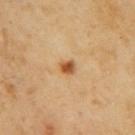Assessment: Imaged during a routine full-body skin examination; the lesion was not biopsied and no histopathology is available. Image and clinical context: A lesion tile, about 15 mm wide, cut from a 3D total-body photograph. The lesion's longest dimension is about 2 mm. Automated image analysis of the tile measured a classifier nevus-likeness of about 95/100 and a lesion-detection confidence of about 100/100. The lesion is on the right upper arm. The tile uses cross-polarized illumination. The patient is a female roughly 40 years of age.